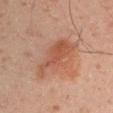notes = no biopsy performed (imaged during a skin exam).